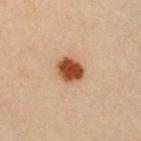This lesion was catalogued during total-body skin photography and was not selected for biopsy. A female patient, approximately 40 years of age. Measured at roughly 3 mm in maximum diameter. On the front of the torso. Captured under cross-polarized illumination. Cropped from a whole-body photographic skin survey; the tile spans about 15 mm.